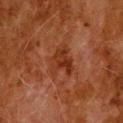{
  "biopsy_status": "not biopsied; imaged during a skin examination",
  "patient": {
    "sex": "male",
    "age_approx": 80
  },
  "image": {
    "source": "total-body photography crop",
    "field_of_view_mm": 15
  },
  "site": "upper back"
}